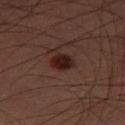No biopsy was performed on this lesion — it was imaged during a full skin examination and was not determined to be concerning. A male subject aged approximately 60. The lesion is located on the right thigh. A roughly 15 mm field-of-view crop from a total-body skin photograph. The lesion-visualizer software estimated a classifier nevus-likeness of about 95/100.